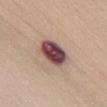The lesion was tiled from a total-body skin photograph and was not biopsied. A region of skin cropped from a whole-body photographic capture, roughly 15 mm wide. Automated image analysis of the tile measured lesion-presence confidence of about 100/100. The lesion is located on the abdomen. The patient is a male roughly 65 years of age. The tile uses white-light illumination.